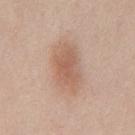Impression:
This lesion was catalogued during total-body skin photography and was not selected for biopsy.
Acquisition and patient details:
About 6 mm across. The lesion is located on the mid back. A 15 mm close-up tile from a total-body photography series done for melanoma screening. Captured under white-light illumination. A male subject aged approximately 60. Automated image analysis of the tile measured border irregularity of about 2 on a 0–10 scale, internal color variation of about 3.5 on a 0–10 scale, and peripheral color asymmetry of about 1. The analysis additionally found a lesion-detection confidence of about 100/100.